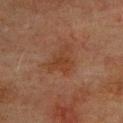follow-up: imaged on a skin check; not biopsied
image: total-body-photography crop, ~15 mm field of view
site: the upper back
lesion diameter: about 4 mm
subject: male, about 75 years old